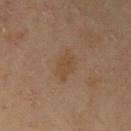Notes:
- workup · no biopsy performed (imaged during a skin exam)
- illumination · cross-polarized illumination
- acquisition · 15 mm crop, total-body photography
- site · the arm
- patient · male, aged 43–47
- automated metrics · an average lesion color of about L≈38 a*≈13 b*≈26 (CIELAB), a lesion–skin lightness drop of about 4, and a lesion-to-skin contrast of about 5 (normalized; higher = more distinct)